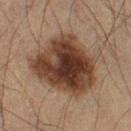biopsy status = catalogued during a skin exam; not biopsied | image = ~15 mm tile from a whole-body skin photo | lesion size = ~8 mm (longest diameter) | TBP lesion metrics = a shape eccentricity near 0.6; a mean CIELAB color near L≈33 a*≈16 b*≈24 and a normalized border contrast of about 12.5; a border-irregularity rating of about 2.5/10, internal color variation of about 7.5 on a 0–10 scale, and a peripheral color-asymmetry measure near 2.5; a nevus-likeness score of about 75/100 and a lesion-detection confidence of about 100/100 | body site = the right thigh | lighting = cross-polarized illumination | patient = male, roughly 55 years of age.